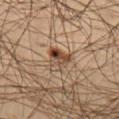notes=catalogued during a skin exam; not biopsied | subject=male, aged around 45 | tile lighting=cross-polarized illumination | site=the left thigh | image=total-body-photography crop, ~15 mm field of view | lesion diameter=≈3.5 mm.